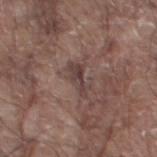Clinical impression: The lesion was photographed on a routine skin check and not biopsied; there is no pathology result. Context: A male subject, aged around 80. The lesion's longest dimension is about 4 mm. Captured under white-light illumination. The lesion is located on the left thigh. Cropped from a total-body skin-imaging series; the visible field is about 15 mm.